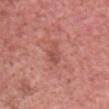| field | value |
|---|---|
| workup | no biopsy performed (imaged during a skin exam) |
| body site | the head or neck |
| image | ~15 mm crop, total-body skin-cancer survey |
| lesion diameter | ≈3 mm |
| subject | male, about 50 years old |